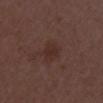biopsy status: catalogued during a skin exam; not biopsied | image-analysis metrics: about 5 CIELAB-L* units darker than the surrounding skin and a normalized border contrast of about 6; a border-irregularity index near 2.5/10; a classifier nevus-likeness of about 5/100 and a detector confidence of about 100 out of 100 that the crop contains a lesion | location: the chest | imaging modality: ~15 mm crop, total-body skin-cancer survey | lesion size: about 2.5 mm | lighting: white-light | patient: female, aged 48 to 52.